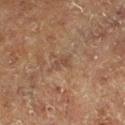No biopsy was performed on this lesion — it was imaged during a full skin examination and was not determined to be concerning.
This image is a 15 mm lesion crop taken from a total-body photograph.
Located on the leg.
This is a cross-polarized tile.
Longest diameter approximately 2.5 mm.
The patient is a male aged 73 to 77.
An algorithmic analysis of the crop reported a mean CIELAB color near L≈37 a*≈15 b*≈23, about 6 CIELAB-L* units darker than the surrounding skin, and a normalized lesion–skin contrast near 5.5. The software also gave a border-irregularity rating of about 3.5/10, a color-variation rating of about 1/10, and a peripheral color-asymmetry measure near 0.5. The software also gave a nevus-likeness score of about 0/100.